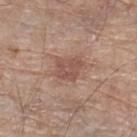<tbp_lesion>
  <patient>
    <sex>male</sex>
    <age_approx>80</age_approx>
  </patient>
  <lighting>white-light</lighting>
  <image>
    <source>total-body photography crop</source>
    <field_of_view_mm>15</field_of_view_mm>
  </image>
  <site>left lower leg</site>
  <lesion_size>
    <long_diameter_mm_approx>3.0</long_diameter_mm_approx>
  </lesion_size>
</tbp_lesion>